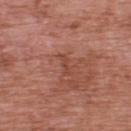Recorded during total-body skin imaging; not selected for excision or biopsy. A male subject aged 68 to 72. A 15 mm crop from a total-body photograph taken for skin-cancer surveillance. An algorithmic analysis of the crop reported a lesion color around L≈46 a*≈26 b*≈29 in CIELAB, roughly 7 lightness units darker than nearby skin, and a lesion-to-skin contrast of about 5 (normalized; higher = more distinct). And it measured a border-irregularity index near 4/10, a color-variation rating of about 0/10, and a peripheral color-asymmetry measure near 0. The lesion is on the upper back.